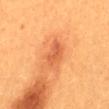Assessment: Part of a total-body skin-imaging series; this lesion was reviewed on a skin check and was not flagged for biopsy. Clinical summary: Imaged with cross-polarized lighting. A roughly 15 mm field-of-view crop from a total-body skin photograph. About 3.5 mm across. On the back. The patient is a female aged approximately 30.